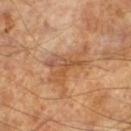Assessment: This lesion was catalogued during total-body skin photography and was not selected for biopsy. Image and clinical context: The tile uses cross-polarized illumination. The lesion-visualizer software estimated a lesion color around L≈53 a*≈22 b*≈35 in CIELAB, a lesion–skin lightness drop of about 8, and a lesion-to-skin contrast of about 6 (normalized; higher = more distinct). The recorded lesion diameter is about 6 mm. A close-up tile cropped from a whole-body skin photograph, about 15 mm across. The subject is a male in their mid- to late 60s.